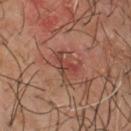Part of a total-body skin-imaging series; this lesion was reviewed on a skin check and was not flagged for biopsy. About 3.5 mm across. From the chest. Cropped from a whole-body photographic skin survey; the tile spans about 15 mm. Imaged with cross-polarized lighting. A male patient, roughly 60 years of age. Automated tile analysis of the lesion measured lesion-presence confidence of about 100/100.